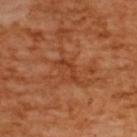notes: no biopsy performed (imaged during a skin exam)
image source: 15 mm crop, total-body photography
subject: female, aged approximately 55
automated metrics: a lesion area of about 3 mm², an eccentricity of roughly 0.95, and two-axis asymmetry of about 0.75; a lesion color around L≈42 a*≈29 b*≈38 in CIELAB, a lesion–skin lightness drop of about 7, and a normalized border contrast of about 6; a border-irregularity rating of about 8/10 and peripheral color asymmetry of about 0
body site: the upper back
lesion diameter: about 3.5 mm
tile lighting: cross-polarized illumination